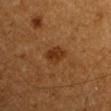Captured during whole-body skin photography for melanoma surveillance; the lesion was not biopsied. On the right upper arm. Automated tile analysis of the lesion measured a classifier nevus-likeness of about 90/100 and a lesion-detection confidence of about 100/100. The recorded lesion diameter is about 3 mm. Imaged with cross-polarized lighting. A male subject, aged 58–62. A 15 mm close-up tile from a total-body photography series done for melanoma screening.